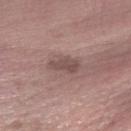The lesion was photographed on a routine skin check and not biopsied; there is no pathology result.
A male subject, aged approximately 60.
This is a white-light tile.
From the right forearm.
About 4 mm across.
A 15 mm close-up extracted from a 3D total-body photography capture.